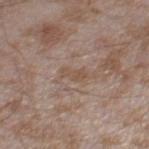Findings:
* workup: total-body-photography surveillance lesion; no biopsy
* illumination: white-light
* anatomic site: the right thigh
* TBP lesion metrics: an average lesion color of about L≈51 a*≈16 b*≈27 (CIELAB) and about 6 CIELAB-L* units darker than the surrounding skin
* subject: male, about 45 years old
* size: ~2.5 mm (longest diameter)
* acquisition: ~15 mm crop, total-body skin-cancer survey